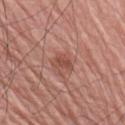Acquisition and patient details: On the right upper arm. This is a white-light tile. Automated tile analysis of the lesion measured a lesion color around L≈49 a*≈24 b*≈27 in CIELAB and about 9 CIELAB-L* units darker than the surrounding skin. About 2.5 mm across. A close-up tile cropped from a whole-body skin photograph, about 15 mm across. The subject is a male in their mid- to late 70s.